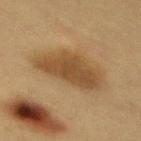Case summary:
* follow-up: imaged on a skin check; not biopsied
* subject: female, aged approximately 40
* site: the mid back
* size: ≈7 mm
* image: 15 mm crop, total-body photography